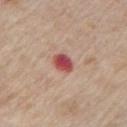| feature | finding |
|---|---|
| workup | total-body-photography surveillance lesion; no biopsy |
| patient | male, about 80 years old |
| acquisition | ~15 mm tile from a whole-body skin photo |
| lesion size | ~3 mm (longest diameter) |
| location | the chest |